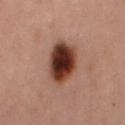<tbp_lesion>
<biopsy_status>not biopsied; imaged during a skin examination</biopsy_status>
</tbp_lesion>